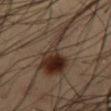biopsy_status: not biopsied; imaged during a skin examination
site: abdomen
automated_metrics:
  nevus_likeness_0_100: 100
  lesion_detection_confidence_0_100: 90
patient:
  sex: male
  age_approx: 55
lighting: cross-polarized
image:
  source: total-body photography crop
  field_of_view_mm: 15
lesion_size:
  long_diameter_mm_approx: 9.5